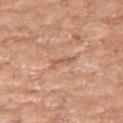Impression:
Part of a total-body skin-imaging series; this lesion was reviewed on a skin check and was not flagged for biopsy.